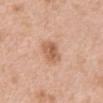{
  "biopsy_status": "not biopsied; imaged during a skin examination",
  "patient": {
    "sex": "female",
    "age_approx": 40
  },
  "site": "abdomen",
  "image": {
    "source": "total-body photography crop",
    "field_of_view_mm": 15
  },
  "automated_metrics": {
    "area_mm2_approx": 6.0,
    "eccentricity": 0.65,
    "nevus_likeness_0_100": 70,
    "lesion_detection_confidence_0_100": 100
  },
  "lesion_size": {
    "long_diameter_mm_approx": 3.0
  }
}